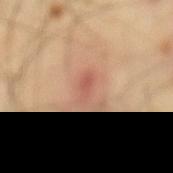workup: imaged on a skin check; not biopsied | subject: male, about 40 years old | diameter: ~3.5 mm (longest diameter) | imaging modality: 15 mm crop, total-body photography | illumination: cross-polarized illumination | location: the mid back.